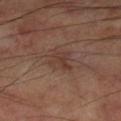No biopsy was performed on this lesion — it was imaged during a full skin examination and was not determined to be concerning. From the left lower leg. A male subject, aged around 70. This is a cross-polarized tile. Cropped from a whole-body photographic skin survey; the tile spans about 15 mm. The recorded lesion diameter is about 3 mm. Automated image analysis of the tile measured a lesion area of about 6 mm² and an eccentricity of roughly 0.4. It also reported a lesion color around L≈36 a*≈17 b*≈23 in CIELAB and a normalized border contrast of about 5.5. And it measured a color-variation rating of about 3/10 and peripheral color asymmetry of about 1. The software also gave a nevus-likeness score of about 0/100 and a lesion-detection confidence of about 100/100.